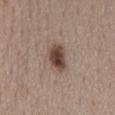- workup · catalogued during a skin exam; not biopsied
- anatomic site · the back
- subject · female, aged 43–47
- imaging modality · total-body-photography crop, ~15 mm field of view
- lighting · white-light
- size · about 4 mm